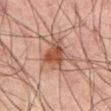Notes:
– workup: imaged on a skin check; not biopsied
– anatomic site: the mid back
– illumination: cross-polarized illumination
– image-analysis metrics: a mean CIELAB color near L≈40 a*≈20 b*≈26, about 10 CIELAB-L* units darker than the surrounding skin, and a normalized border contrast of about 8.5; a border-irregularity index near 4/10, internal color variation of about 4.5 on a 0–10 scale, and a peripheral color-asymmetry measure near 1.5
– lesion size: ~4 mm (longest diameter)
– subject: male, aged 63 to 67
– acquisition: ~15 mm crop, total-body skin-cancer survey